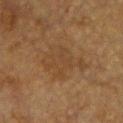{
  "lighting": "cross-polarized",
  "patient": {
    "sex": "female",
    "age_approx": 55
  },
  "lesion_size": {
    "long_diameter_mm_approx": 5.5
  },
  "image": {
    "source": "total-body photography crop",
    "field_of_view_mm": 15
  },
  "site": "chest"
}